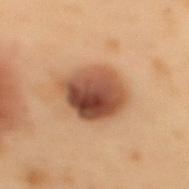Clinical impression:
Recorded during total-body skin imaging; not selected for excision or biopsy.
Background:
A male subject, aged 53 to 57. The lesion is on the mid back. A 15 mm crop from a total-body photograph taken for skin-cancer surveillance. An algorithmic analysis of the crop reported a lesion area of about 19 mm² and a symmetry-axis asymmetry near 0.1. It also reported a lesion color around L≈39 a*≈19 b*≈27 in CIELAB, about 14 CIELAB-L* units darker than the surrounding skin, and a lesion-to-skin contrast of about 11.5 (normalized; higher = more distinct).